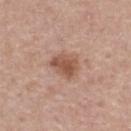Q: Was this lesion biopsied?
A: no biopsy performed (imaged during a skin exam)
Q: What lighting was used for the tile?
A: white-light
Q: What is the lesion's diameter?
A: ≈3.5 mm
Q: Patient demographics?
A: male, in their mid-50s
Q: What kind of image is this?
A: total-body-photography crop, ~15 mm field of view